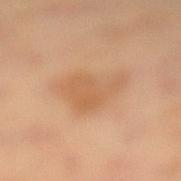workup=no biopsy performed (imaged during a skin exam) | body site=the left lower leg | imaging modality=~15 mm crop, total-body skin-cancer survey | subject=female, aged 63–67.